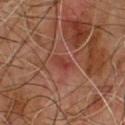Part of a total-body skin-imaging series; this lesion was reviewed on a skin check and was not flagged for biopsy. A region of skin cropped from a whole-body photographic capture, roughly 15 mm wide. The tile uses cross-polarized illumination. The patient is a male aged around 65. The lesion's longest dimension is about 3 mm.